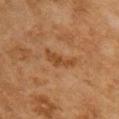Q: Was a biopsy performed?
A: imaged on a skin check; not biopsied
Q: How was the tile lit?
A: cross-polarized
Q: What is the anatomic site?
A: the upper back
Q: Lesion size?
A: ~4.5 mm (longest diameter)
Q: Patient demographics?
A: female, approximately 60 years of age
Q: How was this image acquired?
A: total-body-photography crop, ~15 mm field of view